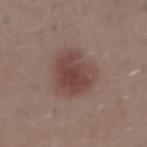The lesion is located on the right upper arm.
The lesion-visualizer software estimated a lesion area of about 18 mm², an eccentricity of roughly 0.55, and a symmetry-axis asymmetry near 0.25. The analysis additionally found an automated nevus-likeness rating near 95 out of 100 and lesion-presence confidence of about 100/100.
A region of skin cropped from a whole-body photographic capture, roughly 15 mm wide.
Imaged with white-light lighting.
A male patient about 30 years old.